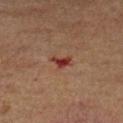Assessment:
This lesion was catalogued during total-body skin photography and was not selected for biopsy.
Background:
A lesion tile, about 15 mm wide, cut from a 3D total-body photograph. Captured under cross-polarized illumination. A male subject roughly 65 years of age. On the left lower leg.